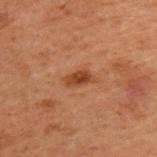Case summary:
• follow-up · total-body-photography surveillance lesion; no biopsy
• patient · male, roughly 60 years of age
• TBP lesion metrics · a within-lesion color-variation index near 2.5/10 and a peripheral color-asymmetry measure near 1; a classifier nevus-likeness of about 90/100 and a detector confidence of about 100 out of 100 that the crop contains a lesion
• lesion size · ~3 mm (longest diameter)
• image source · ~15 mm crop, total-body skin-cancer survey
• body site · the back